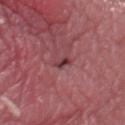Case summary:
• workup: total-body-photography surveillance lesion; no biopsy
• TBP lesion metrics: an eccentricity of roughly 0.95 and two-axis asymmetry of about 0.45; a border-irregularity index near 4.5/10, internal color variation of about 0 on a 0–10 scale, and a peripheral color-asymmetry measure near 0
• lighting: white-light
• image source: ~15 mm crop, total-body skin-cancer survey
• size: about 2.5 mm
• subject: male, aged 38 to 42
• anatomic site: the chest